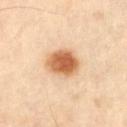follow-up: imaged on a skin check; not biopsied | location: the left thigh | image source: total-body-photography crop, ~15 mm field of view | automated metrics: an eccentricity of roughly 0.5 and a shape-asymmetry score of about 0.15 (0 = symmetric); a lesion–skin lightness drop of about 17 and a lesion-to-skin contrast of about 10.5 (normalized; higher = more distinct); border irregularity of about 1.5 on a 0–10 scale, a color-variation rating of about 5.5/10, and peripheral color asymmetry of about 1.5.